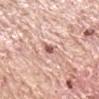Case summary:
* biopsy status · total-body-photography surveillance lesion; no biopsy
* site · the right upper arm
* image source · ~15 mm tile from a whole-body skin photo
* patient · male, in their mid-60s
* illumination · white-light